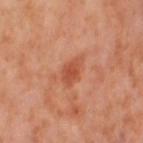– biopsy status — total-body-photography surveillance lesion; no biopsy
– acquisition — 15 mm crop, total-body photography
– patient — female, aged approximately 55
– illumination — cross-polarized illumination
– diameter — ~3.5 mm (longest diameter)
– site — the left thigh
– automated lesion analysis — a footprint of about 5.5 mm², an eccentricity of roughly 0.8, and a symmetry-axis asymmetry near 0.3; a lesion color around L≈53 a*≈30 b*≈36 in CIELAB, a lesion–skin lightness drop of about 9, and a normalized border contrast of about 6.5; a nevus-likeness score of about 40/100 and a lesion-detection confidence of about 100/100